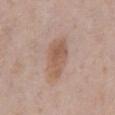Case summary:
– patient — male, aged approximately 70
– lighting — white-light illumination
– lesion diameter — ~5.5 mm (longest diameter)
– site — the chest
– image source — ~15 mm tile from a whole-body skin photo